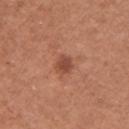notes: total-body-photography surveillance lesion; no biopsy
acquisition: total-body-photography crop, ~15 mm field of view
subject: female, aged 63 to 67
lesion diameter: about 2.5 mm
illumination: white-light
TBP lesion metrics: a lesion-to-skin contrast of about 7 (normalized; higher = more distinct); border irregularity of about 1.5 on a 0–10 scale and radial color variation of about 1; an automated nevus-likeness rating near 40 out of 100 and lesion-presence confidence of about 100/100
site: the left upper arm